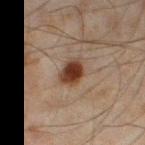site: the right thigh
lighting: cross-polarized illumination
image source: total-body-photography crop, ~15 mm field of view
patient: male, aged 43 to 47
TBP lesion metrics: a symmetry-axis asymmetry near 0.2; roughly 13 lightness units darker than nearby skin and a lesion-to-skin contrast of about 12.5 (normalized; higher = more distinct)